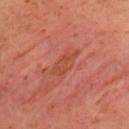{"biopsy_status": "not biopsied; imaged during a skin examination", "patient": {"sex": "female", "age_approx": 40}, "image": {"source": "total-body photography crop", "field_of_view_mm": 15}, "site": "left upper arm", "lighting": "cross-polarized", "lesion_size": {"long_diameter_mm_approx": 4.5}}